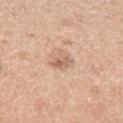image source: total-body-photography crop, ~15 mm field of view; patient: male, in their mid- to late 40s; anatomic site: the left upper arm.